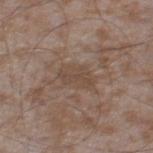workup = catalogued during a skin exam; not biopsied | lesion size = ≈4 mm | automated lesion analysis = an area of roughly 6.5 mm², an eccentricity of roughly 0.85, and two-axis asymmetry of about 0.25; a within-lesion color-variation index near 3/10 and a peripheral color-asymmetry measure near 1; an automated nevus-likeness rating near 0 out of 100 and a detector confidence of about 60 out of 100 that the crop contains a lesion | subject = male, aged around 45 | lighting = white-light | location = the right thigh | image = total-body-photography crop, ~15 mm field of view.